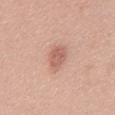patient:
  sex: female
  age_approx: 40
image:
  source: total-body photography crop
  field_of_view_mm: 15
site: chest